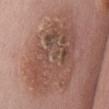workup = no biopsy performed (imaged during a skin exam)
location = the abdomen
imaging modality = ~15 mm tile from a whole-body skin photo
patient = female, aged 83 or older